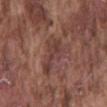biopsy_status: not biopsied; imaged during a skin examination